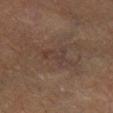biopsy status: total-body-photography surveillance lesion; no biopsy.